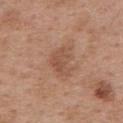workup = no biopsy performed (imaged during a skin exam)